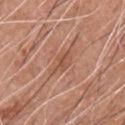Captured during whole-body skin photography for melanoma surveillance; the lesion was not biopsied. From the chest. A roughly 15 mm field-of-view crop from a total-body skin photograph. Automated tile analysis of the lesion measured about 7 CIELAB-L* units darker than the surrounding skin and a lesion-to-skin contrast of about 5.5 (normalized; higher = more distinct). The analysis additionally found border irregularity of about 4 on a 0–10 scale, internal color variation of about 3 on a 0–10 scale, and a peripheral color-asymmetry measure near 1. The software also gave a nevus-likeness score of about 0/100 and a lesion-detection confidence of about 85/100. Imaged with white-light lighting. A male patient, roughly 60 years of age.